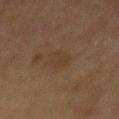subject = male, about 75 years old | imaging modality = ~15 mm crop, total-body skin-cancer survey | anatomic site = the mid back.